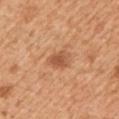follow-up: total-body-photography surveillance lesion; no biopsy | acquisition: 15 mm crop, total-body photography | image-analysis metrics: a border-irregularity index near 2.5/10, a color-variation rating of about 2.5/10, and peripheral color asymmetry of about 1; a nevus-likeness score of about 75/100 and a lesion-detection confidence of about 100/100 | subject: male, about 55 years old | site: the chest.